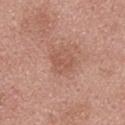Imaged during a routine full-body skin examination; the lesion was not biopsied and no histopathology is available. A close-up tile cropped from a whole-body skin photograph, about 15 mm across. Longest diameter approximately 2.5 mm. The total-body-photography lesion software estimated an area of roughly 4.5 mm² and an outline eccentricity of about 0.7 (0 = round, 1 = elongated). A male subject, aged around 25. Captured under white-light illumination. On the back.